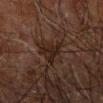Automated image analysis of the tile measured an area of roughly 6.5 mm², an outline eccentricity of about 0.85 (0 = round, 1 = elongated), and a shape-asymmetry score of about 0.5 (0 = symmetric). The analysis additionally found a nevus-likeness score of about 0/100 and lesion-presence confidence of about 55/100.
A lesion tile, about 15 mm wide, cut from a 3D total-body photograph.
The lesion is on the right forearm.
A male subject, approximately 70 years of age.
About 4.5 mm across.
Captured under cross-polarized illumination.